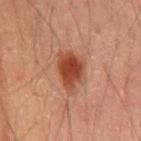Q: Was a biopsy performed?
A: no biopsy performed (imaged during a skin exam)
Q: What lighting was used for the tile?
A: cross-polarized
Q: Automated lesion metrics?
A: an area of roughly 12 mm², an eccentricity of roughly 0.75, and a symmetry-axis asymmetry near 0.2; a lesion color around L≈34 a*≈21 b*≈26 in CIELAB, roughly 10 lightness units darker than nearby skin, and a lesion-to-skin contrast of about 9 (normalized; higher = more distinct); a border-irregularity rating of about 2.5/10 and a peripheral color-asymmetry measure near 1; a nevus-likeness score of about 100/100 and a detector confidence of about 100 out of 100 that the crop contains a lesion
Q: Who is the patient?
A: male, aged approximately 50
Q: What is the imaging modality?
A: ~15 mm tile from a whole-body skin photo
Q: Where on the body is the lesion?
A: the mid back